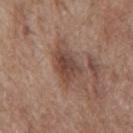{
  "biopsy_status": "not biopsied; imaged during a skin examination",
  "lesion_size": {
    "long_diameter_mm_approx": 4.0
  },
  "site": "mid back",
  "automated_metrics": {
    "border_irregularity_0_10": 2.5,
    "color_variation_0_10": 4.5,
    "peripheral_color_asymmetry": 1.5
  },
  "lighting": "white-light",
  "image": {
    "source": "total-body photography crop",
    "field_of_view_mm": 15
  },
  "patient": {
    "sex": "male",
    "age_approx": 70
  }
}